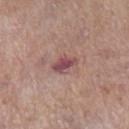biopsy status — no biopsy performed (imaged during a skin exam)
patient — female, aged approximately 85 or older
tile lighting — white-light
image-analysis metrics — a footprint of about 5.5 mm² and a shape-asymmetry score of about 0.25 (0 = symmetric); a border-irregularity index near 2.5/10, internal color variation of about 3.5 on a 0–10 scale, and peripheral color asymmetry of about 1; a lesion-detection confidence of about 100/100
lesion size — ≈3 mm
image source — ~15 mm tile from a whole-body skin photo
anatomic site — the leg